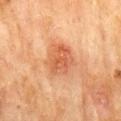Impression:
Recorded during total-body skin imaging; not selected for excision or biopsy.
Clinical summary:
This is a cross-polarized tile. A lesion tile, about 15 mm wide, cut from a 3D total-body photograph. Located on the mid back. A male subject approximately 70 years of age. Automated tile analysis of the lesion measured an area of roughly 10 mm², an outline eccentricity of about 0.5 (0 = round, 1 = elongated), and a symmetry-axis asymmetry near 0.2. It also reported a border-irregularity index near 2/10, a within-lesion color-variation index near 3.5/10, and peripheral color asymmetry of about 1. The software also gave a nevus-likeness score of about 35/100 and a lesion-detection confidence of about 100/100. Longest diameter approximately 4 mm.